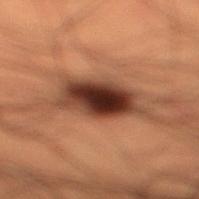No biopsy was performed on this lesion — it was imaged during a full skin examination and was not determined to be concerning.
A 15 mm crop from a total-body photograph taken for skin-cancer surveillance.
Located on the right lower leg.
A male patient, approximately 50 years of age.